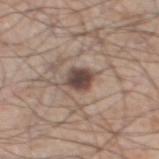Q: Is there a histopathology result?
A: catalogued during a skin exam; not biopsied
Q: What did automated image analysis measure?
A: a mean CIELAB color near L≈46 a*≈15 b*≈21, roughly 15 lightness units darker than nearby skin, and a lesion-to-skin contrast of about 11 (normalized; higher = more distinct); a nevus-likeness score of about 80/100 and a lesion-detection confidence of about 100/100
Q: What is the imaging modality?
A: ~15 mm tile from a whole-body skin photo
Q: Where on the body is the lesion?
A: the left thigh
Q: Lesion size?
A: ≈3.5 mm
Q: What are the patient's age and sex?
A: male, roughly 60 years of age
Q: How was the tile lit?
A: white-light illumination